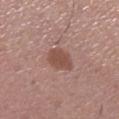Clinical impression:
Imaged during a routine full-body skin examination; the lesion was not biopsied and no histopathology is available.
Acquisition and patient details:
The tile uses white-light illumination. The lesion is on the leg. The lesion-visualizer software estimated a border-irregularity rating of about 1/10 and a peripheral color-asymmetry measure near 0.5. The software also gave a detector confidence of about 100 out of 100 that the crop contains a lesion. Cropped from a total-body skin-imaging series; the visible field is about 15 mm. The patient is a female in their 60s.